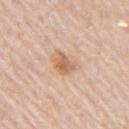Impression: Part of a total-body skin-imaging series; this lesion was reviewed on a skin check and was not flagged for biopsy. Clinical summary: A region of skin cropped from a whole-body photographic capture, roughly 15 mm wide. An algorithmic analysis of the crop reported a footprint of about 4.5 mm², an outline eccentricity of about 0.55 (0 = round, 1 = elongated), and a shape-asymmetry score of about 0.3 (0 = symmetric). It also reported a lesion color around L≈63 a*≈21 b*≈35 in CIELAB and a normalized border contrast of about 7.5. And it measured a color-variation rating of about 3.5/10 and radial color variation of about 1. The analysis additionally found a classifier nevus-likeness of about 40/100 and a lesion-detection confidence of about 100/100. This is a white-light tile. The patient is a male aged 73 to 77. Measured at roughly 2.5 mm in maximum diameter. On the arm.